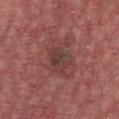The lesion was photographed on a routine skin check and not biopsied; there is no pathology result. A roughly 15 mm field-of-view crop from a total-body skin photograph. A male patient aged 58–62. From the chest.